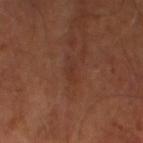• subject — male, in their mid-60s
• image source — 15 mm crop, total-body photography